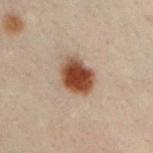workup = no biopsy performed (imaged during a skin exam)
subject = male, aged around 30
imaging modality = 15 mm crop, total-body photography
lighting = cross-polarized illumination
body site = the left upper arm
lesion diameter = about 4 mm